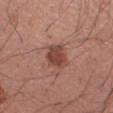Recorded during total-body skin imaging; not selected for excision or biopsy. A male subject aged approximately 35. Imaged with white-light lighting. A 15 mm crop from a total-body photograph taken for skin-cancer surveillance. The lesion-visualizer software estimated a footprint of about 7.5 mm² and a symmetry-axis asymmetry near 0.2. And it measured a mean CIELAB color near L≈45 a*≈24 b*≈26 and roughly 11 lightness units darker than nearby skin. And it measured a border-irregularity index near 2/10, internal color variation of about 4 on a 0–10 scale, and a peripheral color-asymmetry measure near 1.5. The analysis additionally found an automated nevus-likeness rating near 85 out of 100 and lesion-presence confidence of about 100/100. Located on the left forearm.